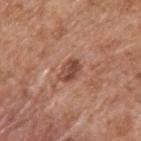Impression:
This lesion was catalogued during total-body skin photography and was not selected for biopsy.
Context:
The patient is a male approximately 65 years of age. The lesion is on the upper back. Cropped from a whole-body photographic skin survey; the tile spans about 15 mm.